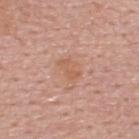follow-up = catalogued during a skin exam; not biopsied
site = the upper back
image-analysis metrics = a classifier nevus-likeness of about 0/100 and a detector confidence of about 100 out of 100 that the crop contains a lesion
subject = male, aged 63–67
acquisition = ~15 mm tile from a whole-body skin photo
illumination = white-light illumination
size = about 3 mm